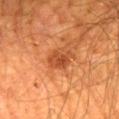Clinical impression: Recorded during total-body skin imaging; not selected for excision or biopsy. Context: Located on the mid back. A male subject roughly 65 years of age. Cropped from a total-body skin-imaging series; the visible field is about 15 mm. The recorded lesion diameter is about 3 mm.